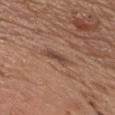| field | value |
|---|---|
| subject | male, about 55 years old |
| automated lesion analysis | an area of roughly 4.5 mm², a shape eccentricity near 0.95, and a symmetry-axis asymmetry near 0.3; internal color variation of about 2.5 on a 0–10 scale |
| size | ~4 mm (longest diameter) |
| tile lighting | white-light illumination |
| imaging modality | total-body-photography crop, ~15 mm field of view |
| site | the chest |